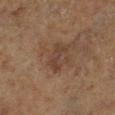Clinical impression: Recorded during total-body skin imaging; not selected for excision or biopsy. Acquisition and patient details: Automated tile analysis of the lesion measured a nevus-likeness score of about 0/100. The patient is a male aged 73–77. On the right lower leg. The tile uses cross-polarized illumination. Cropped from a total-body skin-imaging series; the visible field is about 15 mm.